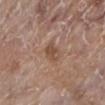Impression:
Recorded during total-body skin imaging; not selected for excision or biopsy.
Background:
Captured under white-light illumination. The lesion is located on the left lower leg. A 15 mm close-up tile from a total-body photography series done for melanoma screening. The subject is a female aged around 85 or older.